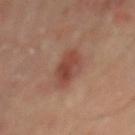Assessment:
The lesion was tiled from a total-body skin photograph and was not biopsied.
Acquisition and patient details:
A male patient, aged 63 to 67. This is a cross-polarized tile. A 15 mm close-up tile from a total-body photography series done for melanoma screening. From the mid back. Approximately 4.5 mm at its widest. Automated tile analysis of the lesion measured a border-irregularity index near 2.5/10, internal color variation of about 3.5 on a 0–10 scale, and peripheral color asymmetry of about 1.5. And it measured a nevus-likeness score of about 95/100.